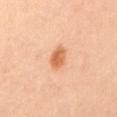The lesion was tiled from a total-body skin photograph and was not biopsied. Measured at roughly 3 mm in maximum diameter. The tile uses cross-polarized illumination. The subject is a female about 50 years old. Cropped from a total-body skin-imaging series; the visible field is about 15 mm.